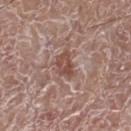lesion size = ≈3.5 mm | imaging modality = total-body-photography crop, ~15 mm field of view | location = the right lower leg | subject = male, aged 73 to 77 | lighting = white-light.